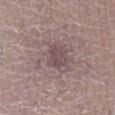workup: no biopsy performed (imaged during a skin exam) | location: the right lower leg | lesion size: ≈2.5 mm | image-analysis metrics: an average lesion color of about L≈47 a*≈18 b*≈13 (CIELAB), a lesion–skin lightness drop of about 8, and a lesion-to-skin contrast of about 6.5 (normalized; higher = more distinct); an automated nevus-likeness rating near 0 out of 100 and lesion-presence confidence of about 95/100 | imaging modality: ~15 mm crop, total-body skin-cancer survey | subject: female, aged approximately 40.